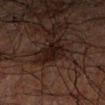Findings:
- follow-up · imaged on a skin check; not biopsied
- image-analysis metrics · a mean CIELAB color near L≈12 a*≈13 b*≈14, a lesion–skin lightness drop of about 7, and a normalized border contrast of about 10; border irregularity of about 3.5 on a 0–10 scale and radial color variation of about 0.5; a nevus-likeness score of about 25/100 and lesion-presence confidence of about 95/100
- size · ≈3 mm
- subject · male, aged around 70
- lighting · cross-polarized illumination
- anatomic site · the right forearm
- imaging modality · ~15 mm tile from a whole-body skin photo